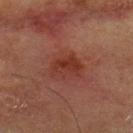  biopsy_status: not biopsied; imaged during a skin examination
  lighting: cross-polarized
  image:
    source: total-body photography crop
    field_of_view_mm: 15
  patient:
    sex: male
    age_approx: 70
  site: back
  lesion_size:
    long_diameter_mm_approx: 3.5
  automated_metrics:
    cielab_L: 28
    cielab_a: 22
    cielab_b: 24
    vs_skin_contrast_norm: 7.0
    border_irregularity_0_10: 3.0
    color_variation_0_10: 3.5
    nevus_likeness_0_100: 15